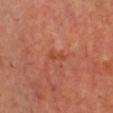{"biopsy_status": "not biopsied; imaged during a skin examination", "site": "chest", "lesion_size": {"long_diameter_mm_approx": 3.0}, "image": {"source": "total-body photography crop", "field_of_view_mm": 15}, "lighting": "cross-polarized", "patient": {"sex": "male", "age_approx": 65}}